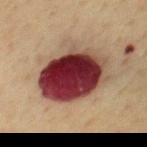biopsy_status: not biopsied; imaged during a skin examination
automated_metrics:
  border_irregularity_0_10: 2.5
  color_variation_0_10: 10.0
  peripheral_color_asymmetry: 4.0
patient:
  sex: male
  age_approx: 60
lighting: cross-polarized
image:
  source: total-body photography crop
  field_of_view_mm: 15
site: chest
lesion_size:
  long_diameter_mm_approx: 7.5